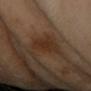biopsy status: no biopsy performed (imaged during a skin exam) | imaging modality: ~15 mm tile from a whole-body skin photo | illumination: cross-polarized illumination | lesion diameter: about 4.5 mm | patient: female, in their 60s | location: the right forearm | image-analysis metrics: a lesion color around L≈30 a*≈17 b*≈27 in CIELAB, roughly 7 lightness units darker than nearby skin, and a lesion-to-skin contrast of about 7.5 (normalized; higher = more distinct); border irregularity of about 3 on a 0–10 scale, internal color variation of about 3.5 on a 0–10 scale, and a peripheral color-asymmetry measure near 1.5; lesion-presence confidence of about 100/100.